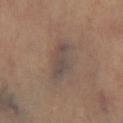{
  "biopsy_status": "not biopsied; imaged during a skin examination",
  "lesion_size": {
    "long_diameter_mm_approx": 4.0
  },
  "lighting": "cross-polarized",
  "image": {
    "source": "total-body photography crop",
    "field_of_view_mm": 15
  },
  "patient": {
    "sex": "female",
    "age_approx": 70
  },
  "site": "right thigh"
}